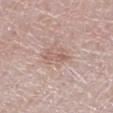Clinical impression: The lesion was tiled from a total-body skin photograph and was not biopsied. Context: The lesion is located on the right lower leg. A female patient, about 55 years old. A roughly 15 mm field-of-view crop from a total-body skin photograph.